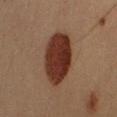<lesion>
  <biopsy_status>not biopsied; imaged during a skin examination</biopsy_status>
  <automated_metrics>
    <area_mm2_approx>20.0</area_mm2_approx>
    <eccentricity>0.75</eccentricity>
    <shape_asymmetry>0.15</shape_asymmetry>
    <border_irregularity_0_10>1.5</border_irregularity_0_10>
    <peripheral_color_asymmetry>1.5</peripheral_color_asymmetry>
  </automated_metrics>
  <lesion_size>
    <long_diameter_mm_approx>6.0</long_diameter_mm_approx>
  </lesion_size>
  <image>
    <source>total-body photography crop</source>
    <field_of_view_mm>15</field_of_view_mm>
  </image>
  <lighting>cross-polarized</lighting>
  <site>right upper arm</site>
  <patient>
    <sex>male</sex>
    <age_approx>40</age_approx>
  </patient>
</lesion>